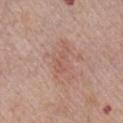Acquisition and patient details: A 15 mm crop from a total-body photograph taken for skin-cancer surveillance. The lesion-visualizer software estimated a lesion color around L≈56 a*≈21 b*≈27 in CIELAB, about 6 CIELAB-L* units darker than the surrounding skin, and a lesion-to-skin contrast of about 4.5 (normalized; higher = more distinct). It also reported a within-lesion color-variation index near 0.5/10 and a peripheral color-asymmetry measure near 0. It also reported a classifier nevus-likeness of about 0/100 and a lesion-detection confidence of about 100/100. From the right upper arm. About 3.5 mm across. A male subject approximately 75 years of age. The tile uses white-light illumination.